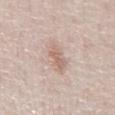This lesion was catalogued during total-body skin photography and was not selected for biopsy. This image is a 15 mm lesion crop taken from a total-body photograph. From the abdomen. A male subject, aged approximately 25.